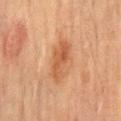workup: no biopsy performed (imaged during a skin exam) | automated lesion analysis: a border-irregularity rating of about 3/10, a color-variation rating of about 3.5/10, and radial color variation of about 1.5 | illumination: cross-polarized | image source: total-body-photography crop, ~15 mm field of view | location: the abdomen | size: ~5 mm (longest diameter) | patient: male, roughly 65 years of age.